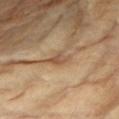The lesion was photographed on a routine skin check and not biopsied; there is no pathology result.
This is a cross-polarized tile.
The lesion-visualizer software estimated about 9 CIELAB-L* units darker than the surrounding skin and a lesion-to-skin contrast of about 6.5 (normalized; higher = more distinct). The software also gave a within-lesion color-variation index near 2.5/10 and radial color variation of about 0.5. It also reported a nevus-likeness score of about 0/100.
Approximately 2.5 mm at its widest.
A female patient aged around 75.
From the left upper arm.
Cropped from a whole-body photographic skin survey; the tile spans about 15 mm.